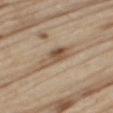follow-up: imaged on a skin check; not biopsied
diameter: about 5 mm
patient: male, aged approximately 70
image source: total-body-photography crop, ~15 mm field of view
anatomic site: the right thigh
TBP lesion metrics: an area of roughly 8 mm², an outline eccentricity of about 0.9 (0 = round, 1 = elongated), and two-axis asymmetry of about 0.3; a border-irregularity rating of about 3.5/10, a color-variation rating of about 7/10, and a peripheral color-asymmetry measure near 2.5; an automated nevus-likeness rating near 40 out of 100 and a lesion-detection confidence of about 90/100